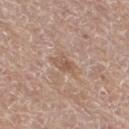Impression:
Imaged during a routine full-body skin examination; the lesion was not biopsied and no histopathology is available.
Acquisition and patient details:
The lesion is located on the right thigh. A female patient, about 75 years old. Captured under white-light illumination. A close-up tile cropped from a whole-body skin photograph, about 15 mm across. Approximately 2.5 mm at its widest.